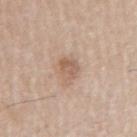Impression: Captured during whole-body skin photography for melanoma surveillance; the lesion was not biopsied. Acquisition and patient details: On the right upper arm. The recorded lesion diameter is about 3 mm. The total-body-photography lesion software estimated an outline eccentricity of about 0.8 (0 = round, 1 = elongated) and two-axis asymmetry of about 0.3. And it measured a lesion color around L≈59 a*≈18 b*≈29 in CIELAB and roughly 9 lightness units darker than nearby skin. The analysis additionally found a border-irregularity index near 3/10. And it measured lesion-presence confidence of about 100/100. The patient is a male roughly 65 years of age. A 15 mm close-up tile from a total-body photography series done for melanoma screening. Imaged with white-light lighting.